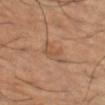{"biopsy_status": "not biopsied; imaged during a skin examination", "patient": {"sex": "male", "age_approx": 65}, "lighting": "cross-polarized", "image": {"source": "total-body photography crop", "field_of_view_mm": 15}, "site": "left thigh", "lesion_size": {"long_diameter_mm_approx": 3.0}}